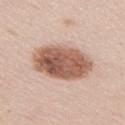Part of a total-body skin-imaging series; this lesion was reviewed on a skin check and was not flagged for biopsy.
A 15 mm close-up tile from a total-body photography series done for melanoma screening.
Longest diameter approximately 6.5 mm.
The total-body-photography lesion software estimated a lesion color around L≈58 a*≈21 b*≈28 in CIELAB and a lesion-to-skin contrast of about 10.5 (normalized; higher = more distinct). And it measured a lesion-detection confidence of about 100/100.
The lesion is on the right upper arm.
This is a white-light tile.
The patient is a female aged 48 to 52.